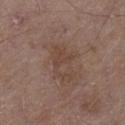follow-up: no biopsy performed (imaged during a skin exam) | imaging modality: ~15 mm crop, total-body skin-cancer survey | subject: male, aged 78–82 | lesion size: about 4 mm | TBP lesion metrics: an outline eccentricity of about 0.75 (0 = round, 1 = elongated) and a symmetry-axis asymmetry near 0.6; an average lesion color of about L≈43 a*≈17 b*≈25 (CIELAB) and a lesion-to-skin contrast of about 5 (normalized; higher = more distinct) | illumination: white-light illumination | site: the left thigh.